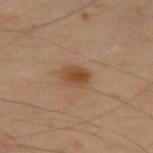Clinical summary:
The subject is a male roughly 70 years of age. On the left thigh. A 15 mm close-up tile from a total-body photography series done for melanoma screening. Captured under cross-polarized illumination.